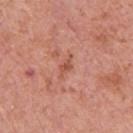Q: Was a biopsy performed?
A: catalogued during a skin exam; not biopsied
Q: Lesion location?
A: the upper back
Q: Lesion size?
A: ~3 mm (longest diameter)
Q: Who is the patient?
A: male, in their 70s
Q: What is the imaging modality?
A: 15 mm crop, total-body photography
Q: How was the tile lit?
A: white-light